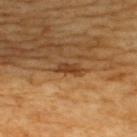Notes:
• biopsy status · total-body-photography surveillance lesion; no biopsy
• image source · ~15 mm tile from a whole-body skin photo
• site · the upper back
• lighting · cross-polarized
• patient · male, aged 58 to 62